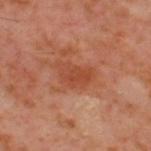Notes:
* automated metrics · border irregularity of about 3 on a 0–10 scale, internal color variation of about 3.5 on a 0–10 scale, and a peripheral color-asymmetry measure near 1
* tile lighting · cross-polarized
* acquisition · 15 mm crop, total-body photography
* subject · male, approximately 60 years of age
* lesion size · about 4.5 mm
* body site · the upper back